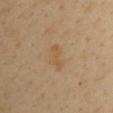Clinical impression: The lesion was photographed on a routine skin check and not biopsied; there is no pathology result. Background: Cropped from a whole-body photographic skin survey; the tile spans about 15 mm. The lesion is located on the right upper arm. The subject is a male roughly 40 years of age. Longest diameter approximately 3 mm. Captured under cross-polarized illumination. Automated tile analysis of the lesion measured a lesion area of about 3 mm², an eccentricity of roughly 0.95, and a symmetry-axis asymmetry near 0.45. It also reported an automated nevus-likeness rating near 0 out of 100.